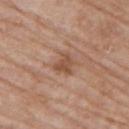Recorded during total-body skin imaging; not selected for excision or biopsy. The total-body-photography lesion software estimated a border-irregularity index near 3.5/10 and a color-variation rating of about 2/10. And it measured a lesion-detection confidence of about 100/100. A lesion tile, about 15 mm wide, cut from a 3D total-body photograph. This is a white-light tile. From the upper back. A female subject, aged around 75. The recorded lesion diameter is about 2.5 mm.